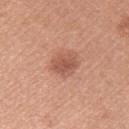notes = imaged on a skin check; not biopsied | acquisition = 15 mm crop, total-body photography | illumination = white-light | size = ≈3 mm | location = the left upper arm | patient = female, roughly 25 years of age.